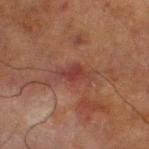Case summary:
* image · ~15 mm crop, total-body skin-cancer survey
* lighting · cross-polarized
* diameter · about 4 mm
* subject · male, about 70 years old
* image-analysis metrics · a classifier nevus-likeness of about 0/100 and a lesion-detection confidence of about 95/100
* anatomic site · the left lower leg